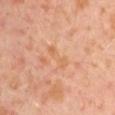Captured during whole-body skin photography for melanoma surveillance; the lesion was not biopsied. Automated tile analysis of the lesion measured a lesion-detection confidence of about 100/100. The tile uses cross-polarized illumination. The lesion is on the left upper arm. A region of skin cropped from a whole-body photographic capture, roughly 15 mm wide. A male subject, approximately 30 years of age.